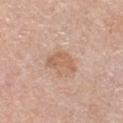Notes:
- follow-up: catalogued during a skin exam; not biopsied
- subject: male, approximately 60 years of age
- lesion size: about 4 mm
- imaging modality: ~15 mm crop, total-body skin-cancer survey
- tile lighting: white-light illumination
- site: the chest
- automated lesion analysis: a lesion area of about 6 mm², an outline eccentricity of about 0.85 (0 = round, 1 = elongated), and a shape-asymmetry score of about 0.45 (0 = symmetric); a mean CIELAB color near L≈61 a*≈20 b*≈32, roughly 8 lightness units darker than nearby skin, and a normalized border contrast of about 6; a border-irregularity rating of about 4.5/10, internal color variation of about 2 on a 0–10 scale, and peripheral color asymmetry of about 0.5; a classifier nevus-likeness of about 0/100 and a detector confidence of about 100 out of 100 that the crop contains a lesion